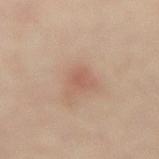| key | value |
|---|---|
| notes | catalogued during a skin exam; not biopsied |
| image | total-body-photography crop, ~15 mm field of view |
| subject | female, aged 63 to 67 |
| site | the abdomen |